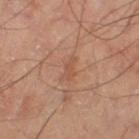Part of a total-body skin-imaging series; this lesion was reviewed on a skin check and was not flagged for biopsy. Located on the leg. Longest diameter approximately 3 mm. Cropped from a whole-body photographic skin survey; the tile spans about 15 mm. A male subject aged around 65.